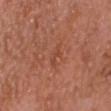Q: Was a biopsy performed?
A: catalogued during a skin exam; not biopsied
Q: Lesion location?
A: the head or neck
Q: Patient demographics?
A: male, approximately 50 years of age
Q: What is the imaging modality?
A: total-body-photography crop, ~15 mm field of view